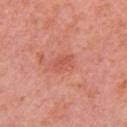{"biopsy_status": "not biopsied; imaged during a skin examination", "lesion_size": {"long_diameter_mm_approx": 2.5}, "site": "arm", "patient": {"sex": "female", "age_approx": 55}, "lighting": "white-light", "image": {"source": "total-body photography crop", "field_of_view_mm": 15}}